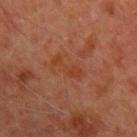follow-up: no biopsy performed (imaged during a skin exam)
tile lighting: cross-polarized illumination
body site: the left upper arm
patient: male, in their mid-60s
image: ~15 mm tile from a whole-body skin photo
image-analysis metrics: an area of roughly 4.5 mm² and an eccentricity of roughly 0.9; an average lesion color of about L≈36 a*≈23 b*≈31 (CIELAB), about 5 CIELAB-L* units darker than the surrounding skin, and a lesion-to-skin contrast of about 6 (normalized; higher = more distinct); a border-irregularity index near 6/10 and a peripheral color-asymmetry measure near 0.5